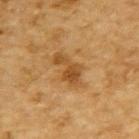Q: Was this lesion biopsied?
A: no biopsy performed (imaged during a skin exam)
Q: How large is the lesion?
A: ~4.5 mm (longest diameter)
Q: Patient demographics?
A: male, in their mid- to late 80s or older
Q: How was this image acquired?
A: 15 mm crop, total-body photography
Q: What did automated image analysis measure?
A: a lesion area of about 8 mm², an outline eccentricity of about 0.85 (0 = round, 1 = elongated), and a shape-asymmetry score of about 0.35 (0 = symmetric); a lesion color around L≈43 a*≈18 b*≈37 in CIELAB, roughly 9 lightness units darker than nearby skin, and a normalized border contrast of about 7.5; a within-lesion color-variation index near 3/10
Q: How was the tile lit?
A: cross-polarized
Q: Lesion location?
A: the back